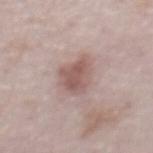subject — male, in their mid-70s
image source — total-body-photography crop, ~15 mm field of view
diameter — ≈4 mm
location — the abdomen
tile lighting — white-light illumination
image-analysis metrics — a footprint of about 9.5 mm² and a shape-asymmetry score of about 0.3 (0 = symmetric); a lesion color around L≈55 a*≈19 b*≈21 in CIELAB, about 11 CIELAB-L* units darker than the surrounding skin, and a normalized lesion–skin contrast near 7.5; internal color variation of about 3 on a 0–10 scale